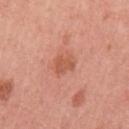anatomic site: the left upper arm
subject: female, approximately 55 years of age
automated lesion analysis: a border-irregularity rating of about 3/10, internal color variation of about 2 on a 0–10 scale, and peripheral color asymmetry of about 0.5; lesion-presence confidence of about 100/100
lesion diameter: about 2.5 mm
tile lighting: white-light
imaging modality: total-body-photography crop, ~15 mm field of view